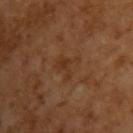Q: Was a biopsy performed?
A: total-body-photography surveillance lesion; no biopsy
Q: How was the tile lit?
A: cross-polarized illumination
Q: Who is the patient?
A: male, aged 63–67
Q: How large is the lesion?
A: about 3.5 mm
Q: How was this image acquired?
A: ~15 mm tile from a whole-body skin photo
Q: What did automated image analysis measure?
A: a lesion color around L≈33 a*≈19 b*≈31 in CIELAB and about 5 CIELAB-L* units darker than the surrounding skin; border irregularity of about 6.5 on a 0–10 scale and a within-lesion color-variation index near 1.5/10; an automated nevus-likeness rating near 0 out of 100